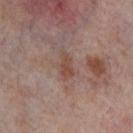Q: Was this lesion biopsied?
A: total-body-photography surveillance lesion; no biopsy
Q: How large is the lesion?
A: about 3.5 mm
Q: What is the imaging modality?
A: ~15 mm tile from a whole-body skin photo
Q: What is the anatomic site?
A: the left thigh
Q: What lighting was used for the tile?
A: cross-polarized illumination
Q: Patient demographics?
A: female, aged approximately 55